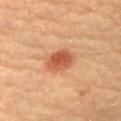Part of a total-body skin-imaging series; this lesion was reviewed on a skin check and was not flagged for biopsy.
The tile uses cross-polarized illumination.
The lesion is on the chest.
A female subject, aged around 60.
Approximately 3.5 mm at its widest.
A roughly 15 mm field-of-view crop from a total-body skin photograph.